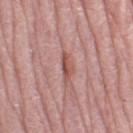Impression:
No biopsy was performed on this lesion — it was imaged during a full skin examination and was not determined to be concerning.
Image and clinical context:
The lesion's longest dimension is about 3.5 mm. A region of skin cropped from a whole-body photographic capture, roughly 15 mm wide. From the leg. A female subject, aged approximately 65. Imaged with white-light lighting.